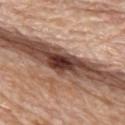On the upper back. This image is a 15 mm lesion crop taken from a total-body photograph. The patient is a female aged 63 to 67.